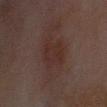No biopsy was performed on this lesion — it was imaged during a full skin examination and was not determined to be concerning. A roughly 15 mm field-of-view crop from a total-body skin photograph. A male subject in their 70s. From the head or neck. The recorded lesion diameter is about 4 mm. Automated image analysis of the tile measured a footprint of about 9 mm² and an eccentricity of roughly 0.8. And it measured a lesion color around L≈22 a*≈15 b*≈16 in CIELAB, a lesion–skin lightness drop of about 4, and a normalized lesion–skin contrast near 5. It also reported a border-irregularity index near 3/10, a within-lesion color-variation index near 2/10, and peripheral color asymmetry of about 0.5.